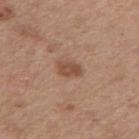Q: Was a biopsy performed?
A: no biopsy performed (imaged during a skin exam)
Q: Patient demographics?
A: male, approximately 75 years of age
Q: What kind of image is this?
A: 15 mm crop, total-body photography
Q: What is the lesion's diameter?
A: ≈3 mm
Q: Lesion location?
A: the right upper arm
Q: Automated lesion metrics?
A: a footprint of about 4 mm² and an eccentricity of roughly 0.8; a lesion color around L≈49 a*≈21 b*≈29 in CIELAB and a lesion–skin lightness drop of about 10; border irregularity of about 2 on a 0–10 scale and a within-lesion color-variation index near 2/10
Q: Illumination type?
A: white-light illumination